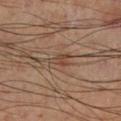Q: Was this lesion biopsied?
A: imaged on a skin check; not biopsied